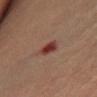The lesion was photographed on a routine skin check and not biopsied; there is no pathology result. Located on the chest. Automated tile analysis of the lesion measured a lesion–skin lightness drop of about 11 and a lesion-to-skin contrast of about 10 (normalized; higher = more distinct). The software also gave a nevus-likeness score of about 0/100. Longest diameter approximately 2.5 mm. A female subject, approximately 80 years of age. The tile uses cross-polarized illumination. A region of skin cropped from a whole-body photographic capture, roughly 15 mm wide.